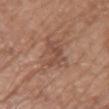This lesion was catalogued during total-body skin photography and was not selected for biopsy. The lesion is on the front of the torso. A female patient approximately 75 years of age. Automated image analysis of the tile measured a lesion area of about 9.5 mm² and a shape-asymmetry score of about 0.4 (0 = symmetric). The analysis additionally found a border-irregularity index near 5.5/10 and a peripheral color-asymmetry measure near 1. The analysis additionally found a nevus-likeness score of about 0/100 and a detector confidence of about 100 out of 100 that the crop contains a lesion. The recorded lesion diameter is about 4 mm. A 15 mm crop from a total-body photograph taken for skin-cancer surveillance.